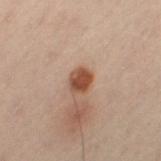Case summary:
- follow-up: no biopsy performed (imaged during a skin exam)
- site: the left thigh
- size: ~2.5 mm (longest diameter)
- image source: 15 mm crop, total-body photography
- subject: male, aged 48 to 52
- illumination: cross-polarized